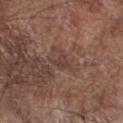{"biopsy_status": "not biopsied; imaged during a skin examination", "site": "right forearm", "lighting": "white-light", "patient": {"sex": "male", "age_approx": 70}, "automated_metrics": {"area_mm2_approx": 3.5, "shape_asymmetry": 0.45, "cielab_L": 40, "cielab_a": 18, "cielab_b": 22, "vs_skin_darker_L": 7.0, "border_irregularity_0_10": 4.5, "color_variation_0_10": 1.5, "peripheral_color_asymmetry": 0.5, "nevus_likeness_0_100": 0, "lesion_detection_confidence_0_100": 85}, "image": {"source": "total-body photography crop", "field_of_view_mm": 15}, "lesion_size": {"long_diameter_mm_approx": 2.5}}